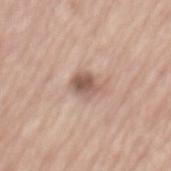<lesion>
  <biopsy_status>not biopsied; imaged during a skin examination</biopsy_status>
  <lighting>white-light</lighting>
  <lesion_size>
    <long_diameter_mm_approx>3.0</long_diameter_mm_approx>
  </lesion_size>
  <patient>
    <sex>male</sex>
    <age_approx>65</age_approx>
  </patient>
  <automated_metrics>
    <eccentricity>0.55</eccentricity>
    <shape_asymmetry>0.2</shape_asymmetry>
    <nevus_likeness_0_100>45</nevus_likeness_0_100>
    <lesion_detection_confidence_0_100>100</lesion_detection_confidence_0_100>
  </automated_metrics>
  <site>mid back</site>
  <image>
    <source>total-body photography crop</source>
    <field_of_view_mm>15</field_of_view_mm>
  </image>
</lesion>